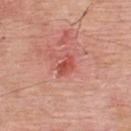Q: Is there a histopathology result?
A: imaged on a skin check; not biopsied
Q: What kind of image is this?
A: ~15 mm tile from a whole-body skin photo
Q: Who is the patient?
A: male, aged 58 to 62
Q: What did automated image analysis measure?
A: about 10 CIELAB-L* units darker than the surrounding skin; a nevus-likeness score of about 25/100
Q: How was the tile lit?
A: white-light
Q: How large is the lesion?
A: ≈3 mm
Q: What is the anatomic site?
A: the upper back